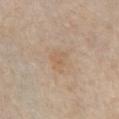The lesion was photographed on a routine skin check and not biopsied; there is no pathology result.
A female patient aged approximately 65.
This image is a 15 mm lesion crop taken from a total-body photograph.
Located on the chest.
The lesion-visualizer software estimated a lesion color around L≈60 a*≈15 b*≈32 in CIELAB, a lesion–skin lightness drop of about 5, and a normalized lesion–skin contrast near 5. It also reported a border-irregularity rating of about 3.5/10, a color-variation rating of about 2.5/10, and peripheral color asymmetry of about 1.
The recorded lesion diameter is about 3 mm.
This is a white-light tile.